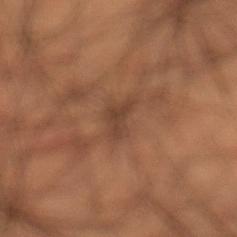The lesion was tiled from a total-body skin photograph and was not biopsied.
The lesion is on the left lower leg.
Automated tile analysis of the lesion measured an area of roughly 3 mm², a shape eccentricity near 0.8, and two-axis asymmetry of about 0.5.
A lesion tile, about 15 mm wide, cut from a 3D total-body photograph.
A male subject, aged 48 to 52.